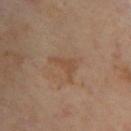biopsy_status: not biopsied; imaged during a skin examination
patient:
  age_approx: 55
lesion_size:
  long_diameter_mm_approx: 3.5
site: upper back
image:
  source: total-body photography crop
  field_of_view_mm: 15